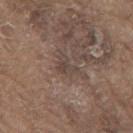  biopsy_status: not biopsied; imaged during a skin examination
  site: mid back
  image:
    source: total-body photography crop
    field_of_view_mm: 15
  patient:
    sex: male
    age_approx: 80
  lesion_size:
    long_diameter_mm_approx: 2.5